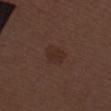Impression:
This lesion was catalogued during total-body skin photography and was not selected for biopsy.
Context:
A male patient aged 68–72. Automated image analysis of the tile measured a lesion color around L≈27 a*≈16 b*≈22 in CIELAB, about 5 CIELAB-L* units darker than the surrounding skin, and a normalized border contrast of about 6. The analysis additionally found border irregularity of about 2 on a 0–10 scale and internal color variation of about 1.5 on a 0–10 scale. And it measured a nevus-likeness score of about 30/100 and a lesion-detection confidence of about 100/100. The lesion is on the leg. A 15 mm close-up extracted from a 3D total-body photography capture.